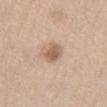TBP lesion metrics — an area of roughly 5.5 mm², an eccentricity of roughly 0.65, and a shape-asymmetry score of about 0.2 (0 = symmetric)
diameter — ~3 mm (longest diameter)
site — the abdomen
illumination — white-light
image — total-body-photography crop, ~15 mm field of view
subject — female, aged 38 to 42